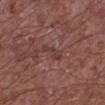follow-up: no biopsy performed (imaged during a skin exam)
tile lighting: white-light
anatomic site: the left lower leg
acquisition: 15 mm crop, total-body photography
patient: male, aged approximately 65
lesion diameter: ≈4 mm
automated lesion analysis: an area of roughly 4 mm², an outline eccentricity of about 0.95 (0 = round, 1 = elongated), and a shape-asymmetry score of about 0.35 (0 = symmetric)